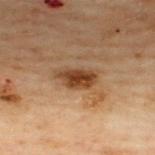Imaged during a routine full-body skin examination; the lesion was not biopsied and no histopathology is available. The tile uses cross-polarized illumination. From the upper back. A male subject about 50 years old. A roughly 15 mm field-of-view crop from a total-body skin photograph.